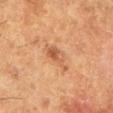Case summary:
- size: ~3.5 mm (longest diameter)
- location: the left lower leg
- imaging modality: ~15 mm crop, total-body skin-cancer survey
- patient: female, aged approximately 50